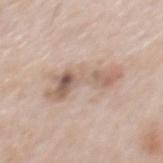{
  "biopsy_status": "not biopsied; imaged during a skin examination",
  "patient": {
    "sex": "male",
    "age_approx": 65
  },
  "lesion_size": {
    "long_diameter_mm_approx": 7.0
  },
  "image": {
    "source": "total-body photography crop",
    "field_of_view_mm": 15
  },
  "site": "mid back",
  "lighting": "white-light"
}